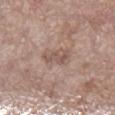- follow-up: no biopsy performed (imaged during a skin exam)
- automated lesion analysis: an average lesion color of about L≈53 a*≈17 b*≈24 (CIELAB), a lesion–skin lightness drop of about 8, and a normalized lesion–skin contrast near 6; border irregularity of about 3.5 on a 0–10 scale, a color-variation rating of about 3/10, and radial color variation of about 1; a detector confidence of about 100 out of 100 that the crop contains a lesion
- lesion diameter: ≈3 mm
- subject: female, aged 83 or older
- body site: the left lower leg
- imaging modality: ~15 mm tile from a whole-body skin photo
- illumination: white-light illumination